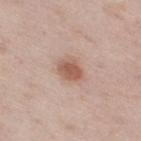The lesion was tiled from a total-body skin photograph and was not biopsied.
A 15 mm close-up tile from a total-body photography series done for melanoma screening.
The patient is a male aged 58–62.
From the right thigh.